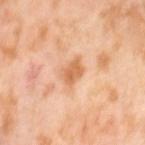Clinical impression: The lesion was tiled from a total-body skin photograph and was not biopsied. Image and clinical context: Located on the left thigh. The lesion-visualizer software estimated roughly 11 lightness units darker than nearby skin and a lesion-to-skin contrast of about 7.5 (normalized; higher = more distinct). It also reported a nevus-likeness score of about 0/100 and lesion-presence confidence of about 100/100. The patient is a female aged around 55. A region of skin cropped from a whole-body photographic capture, roughly 15 mm wide. The lesion's longest dimension is about 3 mm. Captured under cross-polarized illumination.